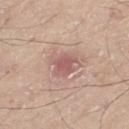Clinical impression: Part of a total-body skin-imaging series; this lesion was reviewed on a skin check and was not flagged for biopsy. Acquisition and patient details: The lesion is on the left thigh. About 3 mm across. A male subject aged 58 to 62. Imaged with white-light lighting. A region of skin cropped from a whole-body photographic capture, roughly 15 mm wide.